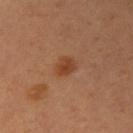follow-up: imaged on a skin check; not biopsied
subject: female, approximately 40 years of age
imaging modality: 15 mm crop, total-body photography
body site: the left arm